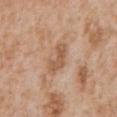| key | value |
|---|---|
| patient | male, about 65 years old |
| size | about 4 mm |
| site | the chest |
| image | total-body-photography crop, ~15 mm field of view |
| illumination | white-light |